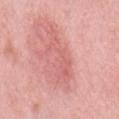<case>
<biopsy_status>not biopsied; imaged during a skin examination</biopsy_status>
<lighting>white-light</lighting>
<lesion_size>
  <long_diameter_mm_approx>7.0</long_diameter_mm_approx>
</lesion_size>
<patient>
  <sex>male</sex>
  <age_approx>55</age_approx>
</patient>
<site>mid back</site>
<image>
  <source>total-body photography crop</source>
  <field_of_view_mm>15</field_of_view_mm>
</image>
</case>